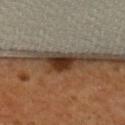Assessment: This lesion was catalogued during total-body skin photography and was not selected for biopsy. Background: Approximately 4.5 mm at its widest. Captured under cross-polarized illumination. Automated tile analysis of the lesion measured a lesion–skin lightness drop of about 17 and a normalized border contrast of about 14. It also reported border irregularity of about 4 on a 0–10 scale and internal color variation of about 4 on a 0–10 scale. It also reported a classifier nevus-likeness of about 100/100. A female patient, aged around 55. A region of skin cropped from a whole-body photographic capture, roughly 15 mm wide. Located on the upper back.